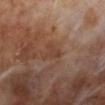Part of a total-body skin-imaging series; this lesion was reviewed on a skin check and was not flagged for biopsy.
A male patient roughly 70 years of age.
A 15 mm close-up extracted from a 3D total-body photography capture.
On the left lower leg.